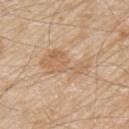Captured during whole-body skin photography for melanoma surveillance; the lesion was not biopsied. A roughly 15 mm field-of-view crop from a total-body skin photograph. This is a white-light tile. An algorithmic analysis of the crop reported a border-irregularity index near 6.5/10, internal color variation of about 3.5 on a 0–10 scale, and a peripheral color-asymmetry measure near 1. The software also gave a nevus-likeness score of about 0/100 and lesion-presence confidence of about 100/100. A male subject in their 80s. On the left upper arm.